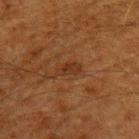Impression:
The lesion was tiled from a total-body skin photograph and was not biopsied.
Image and clinical context:
On the upper back. This is a cross-polarized tile. A lesion tile, about 15 mm wide, cut from a 3D total-body photograph. A male subject aged 58 to 62.